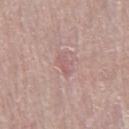• workup — total-body-photography surveillance lesion; no biopsy
• patient — female, approximately 65 years of age
• tile lighting — white-light illumination
• lesion size — ≈3 mm
• acquisition — ~15 mm crop, total-body skin-cancer survey
• body site — the right thigh